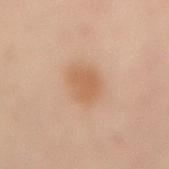{
  "biopsy_status": "not biopsied; imaged during a skin examination",
  "image": {
    "source": "total-body photography crop",
    "field_of_view_mm": 15
  },
  "patient": {
    "sex": "male",
    "age_approx": 40
  },
  "site": "back"
}